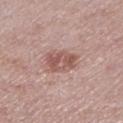Captured during whole-body skin photography for melanoma surveillance; the lesion was not biopsied.